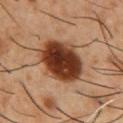This is a cross-polarized tile. A close-up tile cropped from a whole-body skin photograph, about 15 mm across. A male subject, in their mid- to late 50s. On the chest.